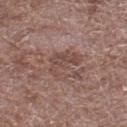{"biopsy_status": "not biopsied; imaged during a skin examination", "site": "left lower leg", "patient": {"sex": "male", "age_approx": 70}, "image": {"source": "total-body photography crop", "field_of_view_mm": 15}, "automated_metrics": {"border_irregularity_0_10": 7.5, "color_variation_0_10": 2.0, "peripheral_color_asymmetry": 0.5}, "lighting": "white-light", "lesion_size": {"long_diameter_mm_approx": 4.0}}